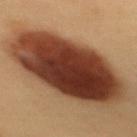The lesion was tiled from a total-body skin photograph and was not biopsied. A 15 mm close-up extracted from a 3D total-body photography capture. The patient is a female aged 28–32. The lesion's longest dimension is about 12 mm. From the upper back.